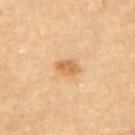<case>
  <biopsy_status>not biopsied; imaged during a skin examination</biopsy_status>
  <image>
    <source>total-body photography crop</source>
    <field_of_view_mm>15</field_of_view_mm>
  </image>
  <patient>
    <sex>male</sex>
    <age_approx>85+</age_approx>
  </patient>
  <automated_metrics>
    <border_irregularity_0_10>2.5</border_irregularity_0_10>
    <color_variation_0_10>2.5</color_variation_0_10>
    <peripheral_color_asymmetry>1.0</peripheral_color_asymmetry>
  </automated_metrics>
  <lighting>cross-polarized</lighting>
  <site>back</site>
  <lesion_size>
    <long_diameter_mm_approx>3.0</long_diameter_mm_approx>
  </lesion_size>
</case>